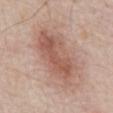Q: Was a biopsy performed?
A: no biopsy performed (imaged during a skin exam)
Q: Who is the patient?
A: male, aged approximately 70
Q: Illumination type?
A: white-light
Q: Where on the body is the lesion?
A: the chest
Q: Lesion size?
A: ≈8 mm
Q: Automated lesion metrics?
A: an area of roughly 24 mm², a shape eccentricity near 0.85, and a symmetry-axis asymmetry near 0.25; a border-irregularity rating of about 3.5/10, a within-lesion color-variation index near 5/10, and peripheral color asymmetry of about 1.5
Q: What is the imaging modality?
A: ~15 mm tile from a whole-body skin photo